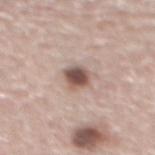The lesion was photographed on a routine skin check and not biopsied; there is no pathology result.
Cropped from a whole-body photographic skin survey; the tile spans about 15 mm.
This is a white-light tile.
About 3 mm across.
A male subject aged 58 to 62.
From the mid back.